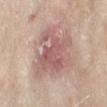<record>
<biopsy_status>not biopsied; imaged during a skin examination</biopsy_status>
<patient>
  <sex>female</sex>
  <age_approx>70</age_approx>
</patient>
<site>left forearm</site>
<automated_metrics>
  <eccentricity>0.55</eccentricity>
  <shape_asymmetry>0.35</shape_asymmetry>
  <cielab_L>58</cielab_L>
  <cielab_a>22</cielab_a>
  <cielab_b>22</cielab_b>
  <vs_skin_darker_L>10.0</vs_skin_darker_L>
  <vs_skin_contrast_norm>6.5</vs_skin_contrast_norm>
  <color_variation_0_10>4.5</color_variation_0_10>
  <peripheral_color_asymmetry>1.5</peripheral_color_asymmetry>
  <nevus_likeness_0_100>0</nevus_likeness_0_100>
  <lesion_detection_confidence_0_100>95</lesion_detection_confidence_0_100>
</automated_metrics>
<lighting>white-light</lighting>
<lesion_size>
  <long_diameter_mm_approx>6.0</long_diameter_mm_approx>
</lesion_size>
<image>
  <source>total-body photography crop</source>
  <field_of_view_mm>15</field_of_view_mm>
</image>
</record>